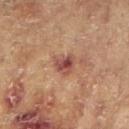– workup · no biopsy performed (imaged during a skin exam)
– subject · female, about 80 years old
– lesion size · ~2.5 mm (longest diameter)
– body site · the left arm
– illumination · cross-polarized
– automated metrics · a lesion color around L≈43 a*≈23 b*≈24 in CIELAB, a lesion–skin lightness drop of about 11, and a normalized border contrast of about 9
– acquisition · ~15 mm tile from a whole-body skin photo